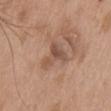Q: Was a biopsy performed?
A: catalogued during a skin exam; not biopsied
Q: Automated lesion metrics?
A: an area of roughly 8 mm², a shape eccentricity near 0.65, and two-axis asymmetry of about 0.6; a border-irregularity index near 6/10, internal color variation of about 5.5 on a 0–10 scale, and a peripheral color-asymmetry measure near 2; a classifier nevus-likeness of about 0/100 and a lesion-detection confidence of about 100/100
Q: How was this image acquired?
A: ~15 mm crop, total-body skin-cancer survey
Q: What lighting was used for the tile?
A: white-light
Q: How large is the lesion?
A: about 4 mm
Q: Patient demographics?
A: male, aged 63–67
Q: Lesion location?
A: the chest